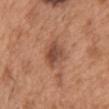Part of a total-body skin-imaging series; this lesion was reviewed on a skin check and was not flagged for biopsy. Imaged with white-light lighting. On the chest. Measured at roughly 3.5 mm in maximum diameter. A close-up tile cropped from a whole-body skin photograph, about 15 mm across. A male patient, roughly 65 years of age. An algorithmic analysis of the crop reported a lesion–skin lightness drop of about 10.